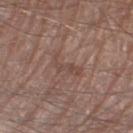Q: Was this lesion biopsied?
A: imaged on a skin check; not biopsied
Q: What kind of image is this?
A: ~15 mm tile from a whole-body skin photo
Q: Lesion location?
A: the right thigh
Q: Who is the patient?
A: male, aged approximately 80
Q: Lesion size?
A: ~3.5 mm (longest diameter)